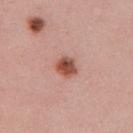{"biopsy_status": "not biopsied; imaged during a skin examination", "site": "right upper arm", "patient": {"sex": "female", "age_approx": 25}, "image": {"source": "total-body photography crop", "field_of_view_mm": 15}}